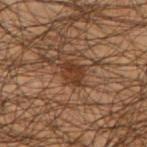Notes:
- workup · total-body-photography surveillance lesion; no biopsy
- acquisition · total-body-photography crop, ~15 mm field of view
- illumination · cross-polarized
- patient · male, aged 48–52
- size · about 3 mm
- body site · the right forearm